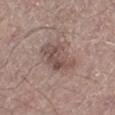Clinical impression:
The lesion was photographed on a routine skin check and not biopsied; there is no pathology result.
Image and clinical context:
The lesion is on the leg. Imaged with white-light lighting. Longest diameter approximately 5 mm. A male patient, approximately 65 years of age. A lesion tile, about 15 mm wide, cut from a 3D total-body photograph. Automated image analysis of the tile measured a lesion area of about 11 mm² and an outline eccentricity of about 0.85 (0 = round, 1 = elongated). And it measured a lesion color around L≈49 a*≈17 b*≈21 in CIELAB, roughly 10 lightness units darker than nearby skin, and a normalized border contrast of about 7. And it measured an automated nevus-likeness rating near 15 out of 100 and lesion-presence confidence of about 100/100.